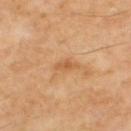Assessment: This lesion was catalogued during total-body skin photography and was not selected for biopsy. Clinical summary: A male subject in their mid-50s. A 15 mm close-up extracted from a 3D total-body photography capture. The tile uses cross-polarized illumination. From the upper back. The lesion-visualizer software estimated an average lesion color of about L≈53 a*≈21 b*≈37 (CIELAB) and a normalized border contrast of about 5.5. The analysis additionally found border irregularity of about 3 on a 0–10 scale, internal color variation of about 1 on a 0–10 scale, and peripheral color asymmetry of about 0.5. It also reported a nevus-likeness score of about 0/100 and a lesion-detection confidence of about 100/100.